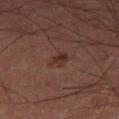The subject is a male aged 58–62. The lesion is on the right thigh. Imaged with cross-polarized lighting. Cropped from a total-body skin-imaging series; the visible field is about 15 mm.